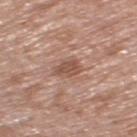Impression: The lesion was tiled from a total-body skin photograph and was not biopsied. Background: The lesion-visualizer software estimated an area of roughly 4.5 mm² and a shape eccentricity near 0.75. It also reported border irregularity of about 2.5 on a 0–10 scale, a within-lesion color-variation index near 1.5/10, and radial color variation of about 0.5. Imaged with white-light lighting. About 3 mm across. A female subject in their mid-70s. Located on the left thigh. A roughly 15 mm field-of-view crop from a total-body skin photograph.